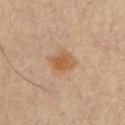automated metrics — a lesion color around L≈46 a*≈16 b*≈30 in CIELAB, about 7 CIELAB-L* units darker than the surrounding skin, and a lesion-to-skin contrast of about 7 (normalized; higher = more distinct); a nevus-likeness score of about 70/100 and a detector confidence of about 100 out of 100 that the crop contains a lesion
imaging modality — 15 mm crop, total-body photography
site — the chest
tile lighting — cross-polarized illumination
patient — male, roughly 60 years of age